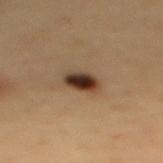{"biopsy_status": "not biopsied; imaged during a skin examination", "patient": {"sex": "male", "age_approx": 55}, "site": "mid back", "image": {"source": "total-body photography crop", "field_of_view_mm": 15}}